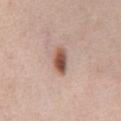biopsy status: imaged on a skin check; not biopsied.